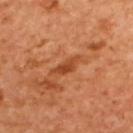biopsy status = total-body-photography surveillance lesion; no biopsy
imaging modality = ~15 mm crop, total-body skin-cancer survey
subject = female, in their mid- to late 50s
lighting = cross-polarized illumination
diameter = ~3.5 mm (longest diameter)
anatomic site = the back